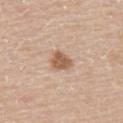follow-up: catalogued during a skin exam; not biopsied.